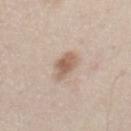– workup · total-body-photography surveillance lesion; no biopsy
– acquisition · ~15 mm tile from a whole-body skin photo
– anatomic site · the front of the torso
– lesion diameter · about 3 mm
– patient · male, in their 60s
– tile lighting · white-light